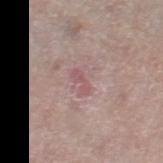biopsy status=total-body-photography surveillance lesion; no biopsy
anatomic site=the right thigh
lesion diameter=≈2.5 mm
subject=female, aged 58 to 62
illumination=white-light
acquisition=~15 mm tile from a whole-body skin photo
automated metrics=an average lesion color of about L≈54 a*≈22 b*≈19 (CIELAB), a lesion–skin lightness drop of about 7, and a normalized border contrast of about 5.5; a border-irregularity rating of about 4/10